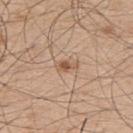Notes:
– follow-up · total-body-photography surveillance lesion; no biopsy
– image · total-body-photography crop, ~15 mm field of view
– image-analysis metrics · a lesion area of about 2.5 mm², an outline eccentricity of about 0.9 (0 = round, 1 = elongated), and a symmetry-axis asymmetry near 0.3; a border-irregularity index near 3/10, a color-variation rating of about 1.5/10, and radial color variation of about 0.5; an automated nevus-likeness rating near 70 out of 100 and a lesion-detection confidence of about 100/100
– illumination · white-light illumination
– patient · male, roughly 50 years of age
– site · the back